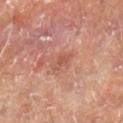Q: What kind of image is this?
A: total-body-photography crop, ~15 mm field of view
Q: Patient demographics?
A: male, aged 68–72
Q: Automated lesion metrics?
A: a nevus-likeness score of about 0/100 and a detector confidence of about 100 out of 100 that the crop contains a lesion
Q: Illumination type?
A: cross-polarized
Q: Where on the body is the lesion?
A: the right lower leg